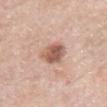The lesion was photographed on a routine skin check and not biopsied; there is no pathology result. This image is a 15 mm lesion crop taken from a total-body photograph. The lesion is on the back. Captured under white-light illumination. A male subject, aged 78 to 82. The lesion's longest dimension is about 3.5 mm.